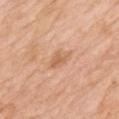Recorded during total-body skin imaging; not selected for excision or biopsy.
A region of skin cropped from a whole-body photographic capture, roughly 15 mm wide.
Captured under white-light illumination.
A female subject roughly 70 years of age.
On the mid back.
The lesion's longest dimension is about 3 mm.